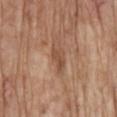Impression:
The lesion was photographed on a routine skin check and not biopsied; there is no pathology result.
Background:
A male subject about 70 years old. Longest diameter approximately 3 mm. A 15 mm close-up extracted from a 3D total-body photography capture. From the mid back. This is a white-light tile.